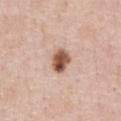Findings:
– workup — total-body-photography surveillance lesion; no biopsy
– image-analysis metrics — a mean CIELAB color near L≈55 a*≈21 b*≈29, roughly 18 lightness units darker than nearby skin, and a lesion-to-skin contrast of about 11.5 (normalized; higher = more distinct); a border-irregularity index near 1.5/10 and a peripheral color-asymmetry measure near 2.5; a classifier nevus-likeness of about 95/100
– subject — male, aged approximately 50
– illumination — white-light illumination
– anatomic site — the abdomen
– imaging modality — ~15 mm tile from a whole-body skin photo